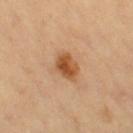This lesion was catalogued during total-body skin photography and was not selected for biopsy. Measured at roughly 3.5 mm in maximum diameter. This is a cross-polarized tile. The lesion is located on the leg. A roughly 15 mm field-of-view crop from a total-body skin photograph. The patient is a female aged 68 to 72.